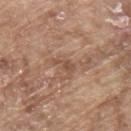automated metrics — a border-irregularity index near 5.5/10, a within-lesion color-variation index near 1/10, and radial color variation of about 0.5
subject — male, aged around 65
lighting — white-light
image — total-body-photography crop, ~15 mm field of view
lesion size — ≈2.5 mm
location — the upper back